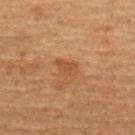<case>
  <biopsy_status>not biopsied; imaged during a skin examination</biopsy_status>
  <site>upper back</site>
  <lesion_size>
    <long_diameter_mm_approx>2.5</long_diameter_mm_approx>
  </lesion_size>
  <lighting>cross-polarized</lighting>
  <image>
    <source>total-body photography crop</source>
    <field_of_view_mm>15</field_of_view_mm>
  </image>
  <patient>
    <sex>female</sex>
    <age_approx>70</age_approx>
  </patient>
</case>